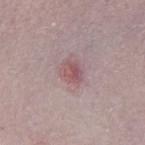Assessment:
This lesion was catalogued during total-body skin photography and was not selected for biopsy.
Clinical summary:
The patient is a female about 60 years old. A 15 mm close-up tile from a total-body photography series done for melanoma screening. On the chest.